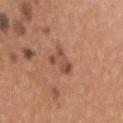Q: How was this image acquired?
A: ~15 mm tile from a whole-body skin photo
Q: Who is the patient?
A: female, aged 18 to 22
Q: How was the tile lit?
A: white-light illumination
Q: Lesion location?
A: the right upper arm
Q: What is the lesion's diameter?
A: ~3.5 mm (longest diameter)